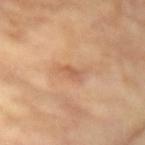workup: no biopsy performed (imaged during a skin exam)
image: ~15 mm tile from a whole-body skin photo
TBP lesion metrics: a lesion color around L≈59 a*≈22 b*≈34 in CIELAB, a lesion–skin lightness drop of about 8, and a lesion-to-skin contrast of about 5.5 (normalized; higher = more distinct); an automated nevus-likeness rating near 0 out of 100 and lesion-presence confidence of about 100/100
body site: the right upper arm
size: ≈3 mm
lighting: cross-polarized illumination
subject: female, aged around 75